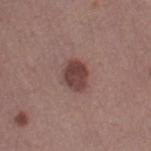follow-up = no biopsy performed (imaged during a skin exam) | image source = ~15 mm crop, total-body skin-cancer survey | TBP lesion metrics = an average lesion color of about L≈41 a*≈20 b*≈21 (CIELAB) and roughly 12 lightness units darker than nearby skin; a border-irregularity rating of about 1.5/10 and a color-variation rating of about 3.5/10; an automated nevus-likeness rating near 75 out of 100 and lesion-presence confidence of about 100/100 | lesion size = ~3.5 mm (longest diameter) | patient = female, aged approximately 50 | body site = the left thigh.